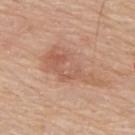| key | value |
|---|---|
| tile lighting | white-light illumination |
| size | ~8 mm (longest diameter) |
| image | total-body-photography crop, ~15 mm field of view |
| subject | male, aged 78–82 |
| site | the upper back |